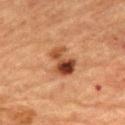The lesion was tiled from a total-body skin photograph and was not biopsied. Longest diameter approximately 4 mm. A region of skin cropped from a whole-body photographic capture, roughly 15 mm wide. The patient is a female aged 68–72. Captured under cross-polarized illumination. Located on the mid back. The lesion-visualizer software estimated an area of roughly 8 mm². It also reported an average lesion color of about L≈39 a*≈23 b*≈32 (CIELAB), a lesion–skin lightness drop of about 13, and a normalized lesion–skin contrast near 10.5. The software also gave a border-irregularity index near 4.5/10, a within-lesion color-variation index near 9.5/10, and a peripheral color-asymmetry measure near 3.5. The software also gave a classifier nevus-likeness of about 75/100 and lesion-presence confidence of about 100/100.